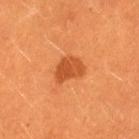Case summary:
• subject — female, aged around 30
• image source — 15 mm crop, total-body photography
• site — the left thigh
• lesion size — ≈3.5 mm
• illumination — cross-polarized